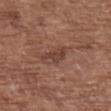No biopsy was performed on this lesion — it was imaged during a full skin examination and was not determined to be concerning. From the upper back. A female patient aged 73–77. Cropped from a whole-body photographic skin survey; the tile spans about 15 mm. The recorded lesion diameter is about 3.5 mm.